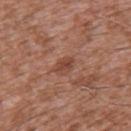The lesion was tiled from a total-body skin photograph and was not biopsied. The lesion is located on the left upper arm. The subject is a male approximately 45 years of age. About 2.5 mm across. Cropped from a total-body skin-imaging series; the visible field is about 15 mm.